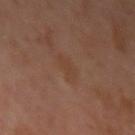{"biopsy_status": "not biopsied; imaged during a skin examination", "automated_metrics": {"eccentricity": 0.8, "shape_asymmetry": 0.35, "border_irregularity_0_10": 4.0, "color_variation_0_10": 1.0, "nevus_likeness_0_100": 0, "lesion_detection_confidence_0_100": 100}, "site": "left upper arm", "patient": {"sex": "male", "age_approx": 60}, "lesion_size": {"long_diameter_mm_approx": 3.0}, "image": {"source": "total-body photography crop", "field_of_view_mm": 15}, "lighting": "cross-polarized"}